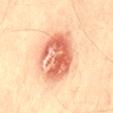Captured during whole-body skin photography for melanoma surveillance; the lesion was not biopsied. Cropped from a total-body skin-imaging series; the visible field is about 15 mm. The lesion is located on the back. A male subject roughly 75 years of age.